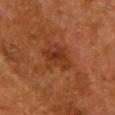| feature | finding |
|---|---|
| follow-up | imaged on a skin check; not biopsied |
| lesion diameter | about 4 mm |
| site | the chest |
| imaging modality | 15 mm crop, total-body photography |
| illumination | cross-polarized illumination |
| patient | female, about 50 years old |